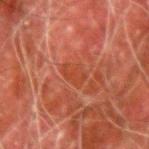  biopsy_status: not biopsied; imaged during a skin examination